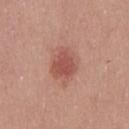Q: Was a biopsy performed?
A: no biopsy performed (imaged during a skin exam)
Q: Who is the patient?
A: male, aged approximately 25
Q: What lighting was used for the tile?
A: white-light
Q: Where on the body is the lesion?
A: the chest
Q: What is the imaging modality?
A: ~15 mm crop, total-body skin-cancer survey